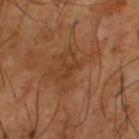* notes: no biopsy performed (imaged during a skin exam)
* image: ~15 mm tile from a whole-body skin photo
* body site: the leg
* patient: male, roughly 65 years of age
* size: ≈2.5 mm